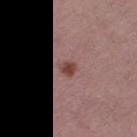The recorded lesion diameter is about 3 mm. The total-body-photography lesion software estimated a border-irregularity index near 3/10 and a color-variation rating of about 4.5/10. A region of skin cropped from a whole-body photographic capture, roughly 15 mm wide. The lesion is on the right thigh. A female patient, in their mid- to late 50s. The tile uses white-light illumination.